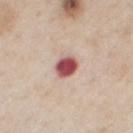biopsy_status: not biopsied; imaged during a skin examination
site: abdomen
lesion_size:
  long_diameter_mm_approx: 3.0
image:
  source: total-body photography crop
  field_of_view_mm: 15
lighting: white-light
patient:
  sex: male
  age_approx: 60